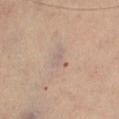Clinical impression: The lesion was tiled from a total-body skin photograph and was not biopsied. Context: Located on the right thigh. Approximately 2.5 mm at its widest. A female subject aged 58–62. This is a cross-polarized tile. A lesion tile, about 15 mm wide, cut from a 3D total-body photograph.